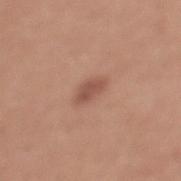Notes:
• notes: imaged on a skin check; not biopsied
• size: ~3 mm (longest diameter)
• body site: the front of the torso
• illumination: white-light illumination
• subject: female, roughly 35 years of age
• image: 15 mm crop, total-body photography